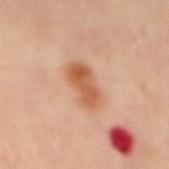  biopsy_status: not biopsied; imaged during a skin examination
  site: mid back
  lighting: cross-polarized
  image:
    source: total-body photography crop
    field_of_view_mm: 15
  patient:
    sex: male
    age_approx: 50
  lesion_size:
    long_diameter_mm_approx: 4.5